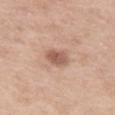Captured during whole-body skin photography for melanoma surveillance; the lesion was not biopsied.
A lesion tile, about 15 mm wide, cut from a 3D total-body photograph.
A female patient, in their mid- to late 50s.
Longest diameter approximately 3 mm.
The lesion is located on the left thigh.